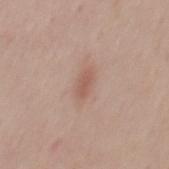{
  "biopsy_status": "not biopsied; imaged during a skin examination",
  "patient": {
    "sex": "male",
    "age_approx": 45
  },
  "image": {
    "source": "total-body photography crop",
    "field_of_view_mm": 15
  },
  "automated_metrics": {
    "cielab_L": 56,
    "cielab_a": 21,
    "cielab_b": 26,
    "vs_skin_darker_L": 8.0,
    "vs_skin_contrast_norm": 6.0
  },
  "lighting": "white-light",
  "site": "mid back",
  "lesion_size": {
    "long_diameter_mm_approx": 3.0
  }
}